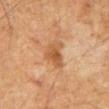Clinical summary: A male subject aged 58 to 62. The lesion's longest dimension is about 4 mm. On the mid back. The tile uses cross-polarized illumination. A close-up tile cropped from a whole-body skin photograph, about 15 mm across.